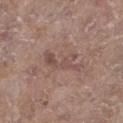This is a white-light tile.
A female subject, aged 83 or older.
The lesion is located on the left lower leg.
A lesion tile, about 15 mm wide, cut from a 3D total-body photograph.